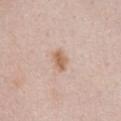Q: Was a biopsy performed?
A: catalogued during a skin exam; not biopsied
Q: What is the imaging modality?
A: ~15 mm tile from a whole-body skin photo
Q: Who is the patient?
A: female, about 50 years old
Q: How large is the lesion?
A: ~3 mm (longest diameter)
Q: Automated lesion metrics?
A: a lesion area of about 4.5 mm², an eccentricity of roughly 0.75, and a symmetry-axis asymmetry near 0.25; a lesion color around L≈63 a*≈19 b*≈31 in CIELAB, roughly 10 lightness units darker than nearby skin, and a normalized border contrast of about 8; a color-variation rating of about 2.5/10 and radial color variation of about 1
Q: How was the tile lit?
A: white-light
Q: Lesion location?
A: the chest